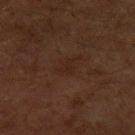biopsy status: no biopsy performed (imaged during a skin exam) | patient: male, roughly 60 years of age | body site: the left upper arm | automated lesion analysis: an outline eccentricity of about 0.75 (0 = round, 1 = elongated) and a shape-asymmetry score of about 0.5 (0 = symmetric); a lesion color around L≈21 a*≈16 b*≈21 in CIELAB, roughly 4 lightness units darker than nearby skin, and a normalized lesion–skin contrast near 5; a border-irregularity index near 5.5/10, a within-lesion color-variation index near 0/10, and a peripheral color-asymmetry measure near 0; an automated nevus-likeness rating near 0 out of 100 and a lesion-detection confidence of about 100/100 | image: total-body-photography crop, ~15 mm field of view | diameter: ~2.5 mm (longest diameter).